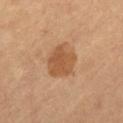<tbp_lesion>
  <biopsy_status>not biopsied; imaged during a skin examination</biopsy_status>
  <lighting>cross-polarized</lighting>
  <image>
    <source>total-body photography crop</source>
    <field_of_view_mm>15</field_of_view_mm>
  </image>
  <site>leg</site>
  <patient>
    <sex>male</sex>
    <age_approx>60</age_approx>
  </patient>
</tbp_lesion>